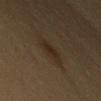Findings:
- biopsy status: no biopsy performed (imaged during a skin exam)
- TBP lesion metrics: a footprint of about 5.5 mm², a shape eccentricity near 0.8, and two-axis asymmetry of about 0.3; a classifier nevus-likeness of about 35/100 and a detector confidence of about 100 out of 100 that the crop contains a lesion
- lesion size: about 3 mm
- subject: female, about 50 years old
- illumination: cross-polarized
- imaging modality: ~15 mm crop, total-body skin-cancer survey
- body site: the left upper arm